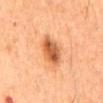Impression:
Part of a total-body skin-imaging series; this lesion was reviewed on a skin check and was not flagged for biopsy.
Context:
A male subject about 65 years old. This is a cross-polarized tile. Automated tile analysis of the lesion measured an average lesion color of about L≈48 a*≈24 b*≈35 (CIELAB), about 13 CIELAB-L* units darker than the surrounding skin, and a lesion-to-skin contrast of about 9 (normalized; higher = more distinct). And it measured a border-irregularity rating of about 2/10, a within-lesion color-variation index near 5/10, and a peripheral color-asymmetry measure near 1.5. A 15 mm close-up tile from a total-body photography series done for melanoma screening. From the mid back. Approximately 4.5 mm at its widest.